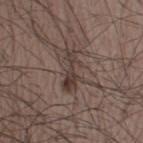biopsy status=no biopsy performed (imaged during a skin exam); image=total-body-photography crop, ~15 mm field of view; patient=male, aged around 65; site=the right thigh; size=≈5 mm.